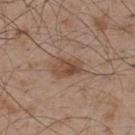Part of a total-body skin-imaging series; this lesion was reviewed on a skin check and was not flagged for biopsy.
A region of skin cropped from a whole-body photographic capture, roughly 15 mm wide.
Measured at roughly 3.5 mm in maximum diameter.
A male patient, about 50 years old.
The lesion is located on the upper back.
Imaged with white-light lighting.